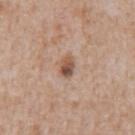This is a white-light tile.
A male patient roughly 65 years of age.
A 15 mm crop from a total-body photograph taken for skin-cancer surveillance.
The total-body-photography lesion software estimated an outline eccentricity of about 0.8 (0 = round, 1 = elongated) and a symmetry-axis asymmetry near 0.3. The software also gave a lesion-to-skin contrast of about 9 (normalized; higher = more distinct). And it measured a border-irregularity index near 2.5/10, internal color variation of about 7 on a 0–10 scale, and peripheral color asymmetry of about 2.5. It also reported a nevus-likeness score of about 80/100 and a detector confidence of about 100 out of 100 that the crop contains a lesion.
The lesion is on the mid back.